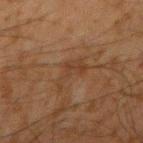Imaged during a routine full-body skin examination; the lesion was not biopsied and no histopathology is available. A 15 mm close-up extracted from a 3D total-body photography capture. Imaged with cross-polarized lighting. The patient is a male aged approximately 35. The lesion is located on the right upper arm. Longest diameter approximately 4 mm.